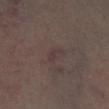The lesion was photographed on a routine skin check and not biopsied; there is no pathology result. From the left lower leg. Approximately 2.5 mm at its widest. A female patient roughly 55 years of age. A lesion tile, about 15 mm wide, cut from a 3D total-body photograph. The tile uses cross-polarized illumination.